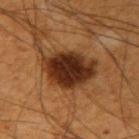This lesion was catalogued during total-body skin photography and was not selected for biopsy.
Approximately 6 mm at its widest.
A 15 mm crop from a total-body photograph taken for skin-cancer surveillance.
The lesion is on the right upper arm.
A male subject aged 63–67.
The lesion-visualizer software estimated a lesion area of about 19 mm², a shape eccentricity near 0.7, and a shape-asymmetry score of about 0.2 (0 = symmetric).
Imaged with cross-polarized lighting.